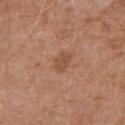  patient:
    sex: male
    age_approx: 75
  image:
    source: total-body photography crop
    field_of_view_mm: 15
  site: chest